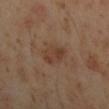| feature | finding |
|---|---|
| follow-up | imaged on a skin check; not biopsied |
| acquisition | ~15 mm crop, total-body skin-cancer survey |
| subject | male, in their mid- to late 40s |
| location | the arm |